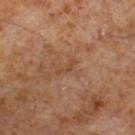Assessment:
Captured during whole-body skin photography for melanoma surveillance; the lesion was not biopsied.
Clinical summary:
The patient is a male aged around 60. The lesion is located on the right lower leg. A 15 mm crop from a total-body photograph taken for skin-cancer surveillance.